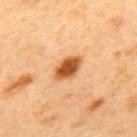- follow-up: total-body-photography surveillance lesion; no biopsy
- subject: male, roughly 50 years of age
- body site: the mid back
- illumination: cross-polarized illumination
- image: total-body-photography crop, ~15 mm field of view